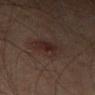The lesion was tiled from a total-body skin photograph and was not biopsied. A male subject approximately 65 years of age. This image is a 15 mm lesion crop taken from a total-body photograph. The lesion is located on the left thigh. The lesion's longest dimension is about 4 mm. The tile uses cross-polarized illumination.